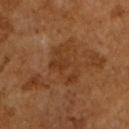Findings:
* notes: catalogued during a skin exam; not biopsied
* patient: male, roughly 65 years of age
* image source: 15 mm crop, total-body photography